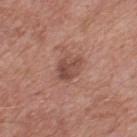follow-up: imaged on a skin check; not biopsied | diameter: ~3.5 mm (longest diameter) | lighting: white-light | site: the upper back | automated metrics: a lesion color around L≈48 a*≈22 b*≈26 in CIELAB, a lesion–skin lightness drop of about 10, and a normalized lesion–skin contrast near 7; a within-lesion color-variation index near 4/10 and peripheral color asymmetry of about 1.5 | imaging modality: total-body-photography crop, ~15 mm field of view | patient: male, in their mid- to late 50s.